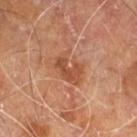biopsy status=no biopsy performed (imaged during a skin exam) | imaging modality=total-body-photography crop, ~15 mm field of view | TBP lesion metrics=an automated nevus-likeness rating near 25 out of 100 and a lesion-detection confidence of about 100/100 | subject=male, roughly 60 years of age | site=the right leg | lighting=cross-polarized illumination | size=≈4 mm.